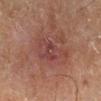{
  "biopsy_status": "not biopsied; imaged during a skin examination",
  "image": {
    "source": "total-body photography crop",
    "field_of_view_mm": 15
  },
  "patient": {
    "sex": "male",
    "age_approx": 65
  },
  "automated_metrics": {
    "vs_skin_contrast_norm": 6.0,
    "border_irregularity_0_10": 5.0,
    "color_variation_0_10": 3.5,
    "peripheral_color_asymmetry": 1.0,
    "nevus_likeness_0_100": 0,
    "lesion_detection_confidence_0_100": 100
  },
  "lighting": "cross-polarized",
  "site": "leg",
  "lesion_size": {
    "long_diameter_mm_approx": 5.0
  }
}